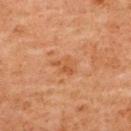Q: Was a biopsy performed?
A: imaged on a skin check; not biopsied
Q: Where on the body is the lesion?
A: the upper back
Q: How large is the lesion?
A: about 3 mm
Q: What kind of image is this?
A: ~15 mm crop, total-body skin-cancer survey
Q: What are the patient's age and sex?
A: female, in their 60s
Q: What lighting was used for the tile?
A: cross-polarized